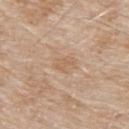Q: Was a biopsy performed?
A: total-body-photography surveillance lesion; no biopsy
Q: What is the anatomic site?
A: the upper back
Q: What kind of image is this?
A: ~15 mm tile from a whole-body skin photo
Q: Patient demographics?
A: male, about 80 years old